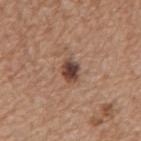Notes:
– notes: catalogued during a skin exam; not biopsied
– patient: male, in their mid- to late 60s
– automated metrics: a border-irregularity index near 2/10, a color-variation rating of about 5/10, and radial color variation of about 1.5; a classifier nevus-likeness of about 90/100 and a detector confidence of about 100 out of 100 that the crop contains a lesion
– tile lighting: white-light illumination
– imaging modality: ~15 mm tile from a whole-body skin photo
– body site: the mid back